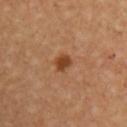workup = no biopsy performed (imaged during a skin exam) | site = the right upper arm | diameter = about 2 mm | image = ~15 mm tile from a whole-body skin photo | patient = female, approximately 45 years of age | tile lighting = cross-polarized.